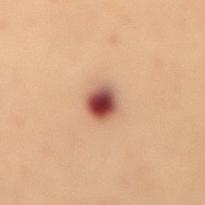The lesion was photographed on a routine skin check and not biopsied; there is no pathology result. This is a cross-polarized tile. The lesion is on the mid back. A female patient aged approximately 50. Automated image analysis of the tile measured a lesion area of about 6 mm², an eccentricity of roughly 0.5, and a shape-asymmetry score of about 0.2 (0 = symmetric). The software also gave a lesion color around L≈43 a*≈23 b*≈25 in CIELAB and a lesion-to-skin contrast of about 13.5 (normalized; higher = more distinct). The analysis additionally found a border-irregularity index near 1.5/10, a within-lesion color-variation index near 8.5/10, and a peripheral color-asymmetry measure near 2.5. The analysis additionally found a nevus-likeness score of about 75/100 and a lesion-detection confidence of about 100/100. Cropped from a total-body skin-imaging series; the visible field is about 15 mm.